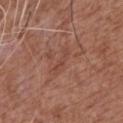The lesion was photographed on a routine skin check and not biopsied; there is no pathology result. A roughly 15 mm field-of-view crop from a total-body skin photograph. Measured at roughly 3 mm in maximum diameter. This is a white-light tile. Located on the chest. A male patient in their mid-70s.